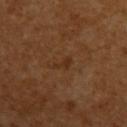Imaged during a routine full-body skin examination; the lesion was not biopsied and no histopathology is available. This is a cross-polarized tile. The recorded lesion diameter is about 2.5 mm. A close-up tile cropped from a whole-body skin photograph, about 15 mm across. A female subject in their mid-50s. The lesion is on the left upper arm.